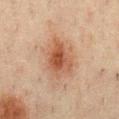Part of a total-body skin-imaging series; this lesion was reviewed on a skin check and was not flagged for biopsy. A male subject aged 48–52. The lesion's longest dimension is about 5 mm. Imaged with cross-polarized lighting. A 15 mm close-up extracted from a 3D total-body photography capture. On the chest. Automated image analysis of the tile measured an average lesion color of about L≈42 a*≈19 b*≈27 (CIELAB), roughly 9 lightness units darker than nearby skin, and a normalized lesion–skin contrast near 8. The analysis additionally found a nevus-likeness score of about 90/100 and lesion-presence confidence of about 100/100.